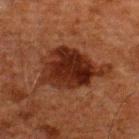Q: What is the lesion's diameter?
A: about 8 mm
Q: Where on the body is the lesion?
A: the upper back
Q: How was this image acquired?
A: 15 mm crop, total-body photography
Q: What are the patient's age and sex?
A: male, approximately 60 years of age
Q: What did automated image analysis measure?
A: a lesion area of about 24 mm² and two-axis asymmetry of about 0.3; a within-lesion color-variation index near 5/10 and peripheral color asymmetry of about 1.5